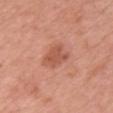Case summary:
- follow-up · catalogued during a skin exam; not biopsied
- size · about 3.5 mm
- patient · female, in their 40s
- acquisition · ~15 mm tile from a whole-body skin photo
- body site · the front of the torso
- tile lighting · white-light